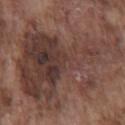Captured during whole-body skin photography for melanoma surveillance; the lesion was not biopsied.
About 14.5 mm across.
The total-body-photography lesion software estimated a footprint of about 85 mm², an eccentricity of roughly 0.8, and a shape-asymmetry score of about 0.45 (0 = symmetric). The software also gave a lesion color around L≈39 a*≈18 b*≈22 in CIELAB and a lesion-to-skin contrast of about 8 (normalized; higher = more distinct). The software also gave lesion-presence confidence of about 60/100.
Cropped from a whole-body photographic skin survey; the tile spans about 15 mm.
This is a white-light tile.
A male subject aged around 75.
On the chest.